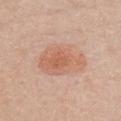The lesion was photographed on a routine skin check and not biopsied; there is no pathology result.
The lesion is on the chest.
A 15 mm crop from a total-body photograph taken for skin-cancer surveillance.
The patient is a female aged 43 to 47.
The lesion's longest dimension is about 6 mm.